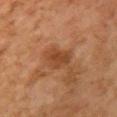Captured during whole-body skin photography for melanoma surveillance; the lesion was not biopsied.
Cropped from a whole-body photographic skin survey; the tile spans about 15 mm.
A female subject in their 60s.
The lesion is located on the front of the torso.